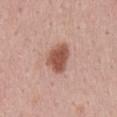{"biopsy_status": "not biopsied; imaged during a skin examination", "lesion_size": {"long_diameter_mm_approx": 4.0}, "site": "mid back", "patient": {"sex": "male", "age_approx": 45}, "image": {"source": "total-body photography crop", "field_of_view_mm": 15}, "lighting": "white-light"}